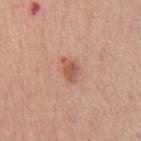Image and clinical context: A close-up tile cropped from a whole-body skin photograph, about 15 mm across. From the chest. The lesion's longest dimension is about 2.5 mm. The subject is a male approximately 55 years of age.